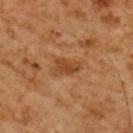Q: Was this lesion biopsied?
A: no biopsy performed (imaged during a skin exam)
Q: Where on the body is the lesion?
A: the upper back
Q: How large is the lesion?
A: ~3 mm (longest diameter)
Q: How was this image acquired?
A: ~15 mm crop, total-body skin-cancer survey
Q: What are the patient's age and sex?
A: male, aged 58 to 62